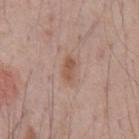Image and clinical context:
The patient is a male aged 68–72. The total-body-photography lesion software estimated a footprint of about 3.5 mm², an eccentricity of roughly 0.9, and two-axis asymmetry of about 0.25. The software also gave a border-irregularity index near 3/10, internal color variation of about 2 on a 0–10 scale, and radial color variation of about 1. Captured under white-light illumination. A 15 mm crop from a total-body photograph taken for skin-cancer surveillance. Measured at roughly 3 mm in maximum diameter. On the chest.